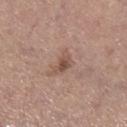Background:
A female subject aged 63–67. Located on the left lower leg. Imaged with white-light lighting. This image is a 15 mm lesion crop taken from a total-body photograph. The total-body-photography lesion software estimated an outline eccentricity of about 0.75 (0 = round, 1 = elongated) and a shape-asymmetry score of about 0.45 (0 = symmetric). The software also gave border irregularity of about 4 on a 0–10 scale, internal color variation of about 2.5 on a 0–10 scale, and peripheral color asymmetry of about 1. The analysis additionally found a classifier nevus-likeness of about 25/100 and a lesion-detection confidence of about 100/100.